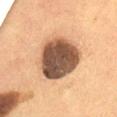The patient is a female about 50 years old. On the abdomen. Cropped from a total-body skin-imaging series; the visible field is about 15 mm.